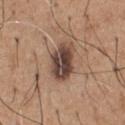biopsy status: no biopsy performed (imaged during a skin exam)
image-analysis metrics: an area of roughly 12 mm², an outline eccentricity of about 0.8 (0 = round, 1 = elongated), and a shape-asymmetry score of about 0.15 (0 = symmetric); a nevus-likeness score of about 35/100 and a detector confidence of about 100 out of 100 that the crop contains a lesion
patient: male, approximately 65 years of age
location: the front of the torso
acquisition: total-body-photography crop, ~15 mm field of view
size: about 4.5 mm